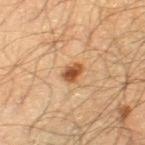workup = catalogued during a skin exam; not biopsied | image = ~15 mm crop, total-body skin-cancer survey | subject = male, approximately 65 years of age | body site = the leg.